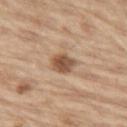- lesion size — about 3 mm
- acquisition — ~15 mm tile from a whole-body skin photo
- body site — the left thigh
- automated metrics — a lesion area of about 6 mm², an eccentricity of roughly 0.65, and two-axis asymmetry of about 0.15
- lighting — white-light illumination
- patient — male, about 70 years old